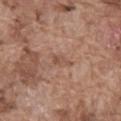Q: Was a biopsy performed?
A: imaged on a skin check; not biopsied
Q: Patient demographics?
A: male, aged 73 to 77
Q: Automated lesion metrics?
A: a footprint of about 3 mm², an eccentricity of roughly 0.9, and a shape-asymmetry score of about 0.35 (0 = symmetric); an average lesion color of about L≈51 a*≈21 b*≈28 (CIELAB), roughly 7 lightness units darker than nearby skin, and a lesion-to-skin contrast of about 5.5 (normalized; higher = more distinct)
Q: What lighting was used for the tile?
A: white-light
Q: What is the imaging modality?
A: ~15 mm tile from a whole-body skin photo
Q: Where on the body is the lesion?
A: the abdomen